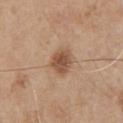This lesion was catalogued during total-body skin photography and was not selected for biopsy. Imaged with white-light lighting. The lesion is on the chest. This image is a 15 mm lesion crop taken from a total-body photograph. Automated image analysis of the tile measured a shape eccentricity near 0.6 and two-axis asymmetry of about 0.2. And it measured a mean CIELAB color near L≈51 a*≈20 b*≈31 and a lesion-to-skin contrast of about 8.5 (normalized; higher = more distinct). The software also gave border irregularity of about 1.5 on a 0–10 scale, a within-lesion color-variation index near 3.5/10, and radial color variation of about 1. A male patient, approximately 65 years of age. Measured at roughly 3.5 mm in maximum diameter.